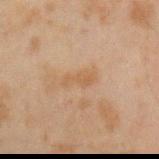Impression: No biopsy was performed on this lesion — it was imaged during a full skin examination and was not determined to be concerning. Image and clinical context: On the left forearm. Cropped from a total-body skin-imaging series; the visible field is about 15 mm. A male subject, aged 43 to 47.